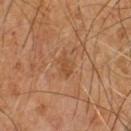Case summary:
- subject — male, roughly 65 years of age
- tile lighting — cross-polarized illumination
- TBP lesion metrics — a border-irregularity rating of about 4/10, internal color variation of about 2 on a 0–10 scale, and radial color variation of about 0.5; a classifier nevus-likeness of about 0/100 and a detector confidence of about 100 out of 100 that the crop contains a lesion
- imaging modality — 15 mm crop, total-body photography